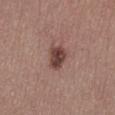<lesion>
<image>
  <source>total-body photography crop</source>
  <field_of_view_mm>15</field_of_view_mm>
</image>
<lesion_size>
  <long_diameter_mm_approx>3.5</long_diameter_mm_approx>
</lesion_size>
<site>chest</site>
<patient>
  <sex>male</sex>
  <age_approx>40</age_approx>
</patient>
<lighting>white-light</lighting>
</lesion>